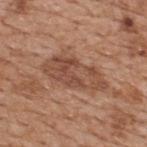Assessment: The lesion was photographed on a routine skin check and not biopsied; there is no pathology result. Acquisition and patient details: The subject is a male aged 63 to 67. A 15 mm close-up extracted from a 3D total-body photography capture. On the upper back.